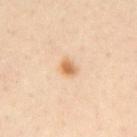<record>
<biopsy_status>not biopsied; imaged during a skin examination</biopsy_status>
<site>chest</site>
<automated_metrics>
  <eccentricity>0.6</eccentricity>
  <shape_asymmetry>0.3</shape_asymmetry>
</automated_metrics>
<image>
  <source>total-body photography crop</source>
  <field_of_view_mm>15</field_of_view_mm>
</image>
<lighting>cross-polarized</lighting>
<patient>
  <sex>male</sex>
  <age_approx>30</age_approx>
</patient>
<lesion_size>
  <long_diameter_mm_approx>2.5</long_diameter_mm_approx>
</lesion_size>
</record>